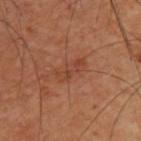This lesion was catalogued during total-body skin photography and was not selected for biopsy. The recorded lesion diameter is about 4 mm. This image is a 15 mm lesion crop taken from a total-body photograph. Located on the upper back. This is a cross-polarized tile. A male patient, in their 60s.